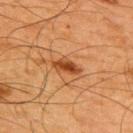Part of a total-body skin-imaging series; this lesion was reviewed on a skin check and was not flagged for biopsy. Automated tile analysis of the lesion measured a border-irregularity rating of about 3/10, a within-lesion color-variation index near 4.5/10, and peripheral color asymmetry of about 1.5. It also reported a lesion-detection confidence of about 100/100. The lesion is on the upper back. Measured at roughly 3.5 mm in maximum diameter. The subject is a male in their mid-60s. A roughly 15 mm field-of-view crop from a total-body skin photograph.